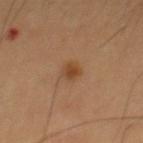Longest diameter approximately 2.5 mm. The patient is a male roughly 60 years of age. A lesion tile, about 15 mm wide, cut from a 3D total-body photograph. Located on the chest. The tile uses cross-polarized illumination. The lesion-visualizer software estimated a lesion color around L≈42 a*≈19 b*≈33 in CIELAB, about 8 CIELAB-L* units darker than the surrounding skin, and a lesion-to-skin contrast of about 7.5 (normalized; higher = more distinct).